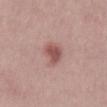– biopsy status: catalogued during a skin exam; not biopsied
– subject: male, aged around 30
– lighting: white-light
– image source: ~15 mm crop, total-body skin-cancer survey